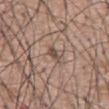Q: Was this lesion biopsied?
A: imaged on a skin check; not biopsied
Q: Where on the body is the lesion?
A: the abdomen
Q: How was this image acquired?
A: ~15 mm crop, total-body skin-cancer survey
Q: How large is the lesion?
A: about 2.5 mm
Q: Patient demographics?
A: male, aged around 60
Q: How was the tile lit?
A: white-light illumination
Q: Automated lesion metrics?
A: a mean CIELAB color near L≈49 a*≈16 b*≈23, roughly 11 lightness units darker than nearby skin, and a normalized lesion–skin contrast near 8; a border-irregularity index near 3.5/10, internal color variation of about 0 on a 0–10 scale, and peripheral color asymmetry of about 0; a detector confidence of about 90 out of 100 that the crop contains a lesion